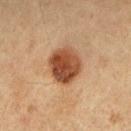{"biopsy_status": "not biopsied; imaged during a skin examination", "automated_metrics": {"border_irregularity_0_10": 1.5, "color_variation_0_10": 6.5, "peripheral_color_asymmetry": 2.5}, "site": "right forearm", "patient": {"sex": "female", "age_approx": 45}, "image": {"source": "total-body photography crop", "field_of_view_mm": 15}, "lesion_size": {"long_diameter_mm_approx": 5.0}}